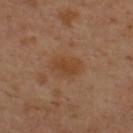{
  "biopsy_status": "not biopsied; imaged during a skin examination",
  "patient": {
    "sex": "male",
    "age_approx": 55
  },
  "site": "upper back",
  "lighting": "cross-polarized",
  "automated_metrics": {
    "lesion_detection_confidence_0_100": 100
  },
  "lesion_size": {
    "long_diameter_mm_approx": 3.5
  },
  "image": {
    "source": "total-body photography crop",
    "field_of_view_mm": 15
  }
}